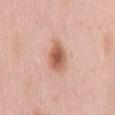notes: no biopsy performed (imaged during a skin exam)
anatomic site: the mid back
image source: 15 mm crop, total-body photography
tile lighting: white-light illumination
subject: female, aged 48–52
lesion diameter: about 4 mm